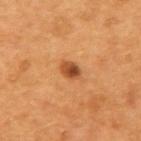{"site": "left upper arm", "image": {"source": "total-body photography crop", "field_of_view_mm": 15}, "patient": {"sex": "male", "age_approx": 55}}